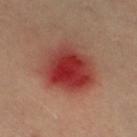site — the right thigh
subject — female, approximately 40 years of age
lesion diameter — ~6 mm (longest diameter)
image source — total-body-photography crop, ~15 mm field of view
illumination — cross-polarized